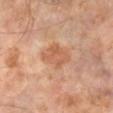biopsy_status: not biopsied; imaged during a skin examination
automated_metrics:
  vs_skin_darker_L: 8.0
  vs_skin_contrast_norm: 6.0
  border_irregularity_0_10: 3.5
  color_variation_0_10: 2.5
  peripheral_color_asymmetry: 1.0
  nevus_likeness_0_100: 0
  lesion_detection_confidence_0_100: 100
lesion_size:
  long_diameter_mm_approx: 4.0
image:
  source: total-body photography crop
  field_of_view_mm: 15
lighting: cross-polarized
patient:
  sex: male
  age_approx: 60
site: left lower leg